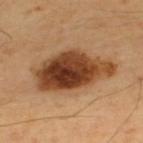notes: no biopsy performed (imaged during a skin exam) | body site: the upper back | illumination: cross-polarized | diameter: about 9 mm | image source: ~15 mm crop, total-body skin-cancer survey | patient: male, in their mid-60s.